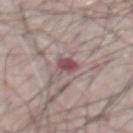Impression: This lesion was catalogued during total-body skin photography and was not selected for biopsy. Background: This is a white-light tile. Located on the abdomen. Measured at roughly 3 mm in maximum diameter. A close-up tile cropped from a whole-body skin photograph, about 15 mm across. A male subject in their mid- to late 60s.